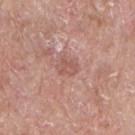| feature | finding |
|---|---|
| follow-up | no biopsy performed (imaged during a skin exam) |
| location | the chest |
| subject | male, aged 63–67 |
| image | ~15 mm crop, total-body skin-cancer survey |
| illumination | white-light illumination |
| image-analysis metrics | a lesion area of about 4 mm², a shape eccentricity near 0.7, and two-axis asymmetry of about 0.35; an average lesion color of about L≈55 a*≈23 b*≈25 (CIELAB), roughly 8 lightness units darker than nearby skin, and a lesion-to-skin contrast of about 5.5 (normalized; higher = more distinct); a border-irregularity index near 3.5/10 and radial color variation of about 1; a classifier nevus-likeness of about 0/100 |